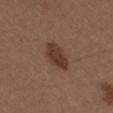This lesion was catalogued during total-body skin photography and was not selected for biopsy.
Approximately 4.5 mm at its widest.
A male subject, aged approximately 30.
Imaged with white-light lighting.
From the right upper arm.
A lesion tile, about 15 mm wide, cut from a 3D total-body photograph.